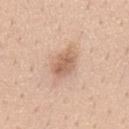This is a white-light tile. Measured at roughly 4 mm in maximum diameter. An algorithmic analysis of the crop reported a lesion area of about 7 mm², an outline eccentricity of about 0.75 (0 = round, 1 = elongated), and a symmetry-axis asymmetry near 0.15. The analysis additionally found an automated nevus-likeness rating near 50 out of 100 and a lesion-detection confidence of about 100/100. A 15 mm crop from a total-body photograph taken for skin-cancer surveillance. A male subject, aged 38–42. The lesion is located on the mid back.